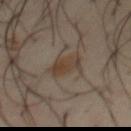{"biopsy_status": "not biopsied; imaged during a skin examination", "site": "chest", "patient": {"sex": "male", "age_approx": 40}, "image": {"source": "total-body photography crop", "field_of_view_mm": 15}}